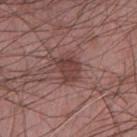Q: What is the imaging modality?
A: 15 mm crop, total-body photography
Q: How was the tile lit?
A: white-light illumination
Q: Lesion size?
A: ≈3 mm
Q: Where on the body is the lesion?
A: the chest
Q: Who is the patient?
A: male, aged 58 to 62